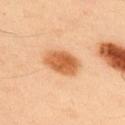Case summary:
* illumination — cross-polarized illumination
* patient — male, roughly 50 years of age
* site — the back
* image source — ~15 mm crop, total-body skin-cancer survey